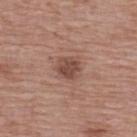| key | value |
|---|---|
| biopsy status | total-body-photography surveillance lesion; no biopsy |
| location | the upper back |
| imaging modality | ~15 mm crop, total-body skin-cancer survey |
| lesion diameter | ≈2.5 mm |
| subject | female, roughly 65 years of age |